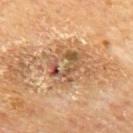Image and clinical context: A male subject, in their mid-60s. The tile uses cross-polarized illumination. On the mid back. A 15 mm close-up tile from a total-body photography series done for melanoma screening.